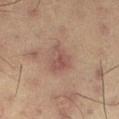The lesion was tiled from a total-body skin photograph and was not biopsied. Measured at roughly 3 mm in maximum diameter. A male patient, in their 60s. The tile uses cross-polarized illumination. This image is a 15 mm lesion crop taken from a total-body photograph. The total-body-photography lesion software estimated a footprint of about 6.5 mm², an outline eccentricity of about 0.55 (0 = round, 1 = elongated), and a shape-asymmetry score of about 0.4 (0 = symmetric). And it measured an average lesion color of about L≈44 a*≈18 b*≈21 (CIELAB) and a lesion–skin lightness drop of about 7. The analysis additionally found a border-irregularity index near 4/10, a color-variation rating of about 3/10, and radial color variation of about 1. The software also gave a nevus-likeness score of about 5/100 and a detector confidence of about 100 out of 100 that the crop contains a lesion.